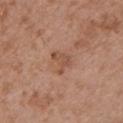biopsy status = total-body-photography surveillance lesion; no biopsy | imaging modality = ~15 mm tile from a whole-body skin photo | illumination = white-light illumination | site = the left upper arm | lesion diameter = about 3 mm | patient = male, aged approximately 50 | automated metrics = roughly 8 lightness units darker than nearby skin and a normalized lesion–skin contrast near 6; a nevus-likeness score of about 0/100 and a detector confidence of about 100 out of 100 that the crop contains a lesion.